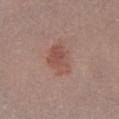Clinical impression:
Recorded during total-body skin imaging; not selected for excision or biopsy.
Background:
A 15 mm crop from a total-body photograph taken for skin-cancer surveillance. The tile uses white-light illumination. The lesion is on the right lower leg. The recorded lesion diameter is about 4 mm. Automated image analysis of the tile measured internal color variation of about 3.5 on a 0–10 scale. The software also gave a nevus-likeness score of about 85/100 and a detector confidence of about 100 out of 100 that the crop contains a lesion. A female patient, about 50 years old.